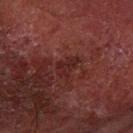Q: Was a biopsy performed?
A: catalogued during a skin exam; not biopsied
Q: What are the patient's age and sex?
A: male, aged 68–72
Q: Lesion location?
A: the right forearm
Q: How was this image acquired?
A: 15 mm crop, total-body photography